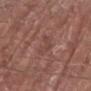| feature | finding |
|---|---|
| follow-up | imaged on a skin check; not biopsied |
| patient | male, aged approximately 80 |
| automated lesion analysis | a nevus-likeness score of about 0/100 |
| location | the arm |
| image source | ~15 mm crop, total-body skin-cancer survey |